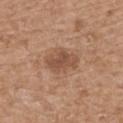Impression:
The lesion was photographed on a routine skin check and not biopsied; there is no pathology result.
Clinical summary:
On the mid back. A close-up tile cropped from a whole-body skin photograph, about 15 mm across. The lesion's longest dimension is about 4 mm. Imaged with white-light lighting. The patient is a male aged 68 to 72.